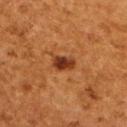Cropped from a total-body skin-imaging series; the visible field is about 15 mm. This is a cross-polarized tile. A female subject, aged around 50. An algorithmic analysis of the crop reported a footprint of about 4.5 mm², a shape eccentricity near 0.8, and two-axis asymmetry of about 0.25. The software also gave an average lesion color of about L≈30 a*≈23 b*≈31 (CIELAB) and roughly 12 lightness units darker than nearby skin. The software also gave an automated nevus-likeness rating near 95 out of 100 and a lesion-detection confidence of about 100/100. Located on the upper back.